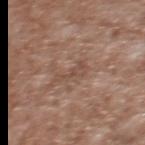Q: Is there a histopathology result?
A: no biopsy performed (imaged during a skin exam)
Q: What kind of image is this?
A: ~15 mm crop, total-body skin-cancer survey
Q: Who is the patient?
A: male, aged around 45
Q: What is the anatomic site?
A: the upper back
Q: What did automated image analysis measure?
A: a within-lesion color-variation index near 1/10 and radial color variation of about 0.5
Q: How large is the lesion?
A: ~3.5 mm (longest diameter)